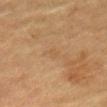| field | value |
|---|---|
| lesion size | about 1.5 mm |
| tile lighting | cross-polarized illumination |
| acquisition | ~15 mm tile from a whole-body skin photo |
| patient | male, in their mid-80s or older |
| anatomic site | the mid back |